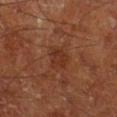Findings:
– follow-up · imaged on a skin check; not biopsied
– subject · male, in their mid- to late 60s
– body site · the right lower leg
– acquisition · 15 mm crop, total-body photography